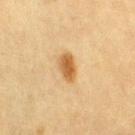biopsy status = catalogued during a skin exam; not biopsied